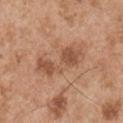| feature | finding |
|---|---|
| biopsy status | no biopsy performed (imaged during a skin exam) |
| tile lighting | white-light illumination |
| image-analysis metrics | a border-irregularity rating of about 5.5/10, a color-variation rating of about 3.5/10, and peripheral color asymmetry of about 1.5 |
| body site | the right upper arm |
| subject | male, aged 53 to 57 |
| image | total-body-photography crop, ~15 mm field of view |